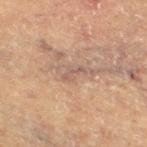Assessment: This lesion was catalogued during total-body skin photography and was not selected for biopsy. Background: Captured under cross-polarized illumination. Located on the right thigh. The subject is a male aged around 65. A 15 mm close-up tile from a total-body photography series done for melanoma screening.